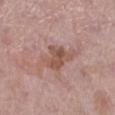The lesion was photographed on a routine skin check and not biopsied; there is no pathology result.
A close-up tile cropped from a whole-body skin photograph, about 15 mm across.
A female patient approximately 70 years of age.
On the left lower leg.
An algorithmic analysis of the crop reported an area of roughly 10 mm², an outline eccentricity of about 0.8 (0 = round, 1 = elongated), and two-axis asymmetry of about 0.3. The software also gave a within-lesion color-variation index near 3.5/10 and a peripheral color-asymmetry measure near 1. And it measured a classifier nevus-likeness of about 0/100 and a lesion-detection confidence of about 100/100.
The lesion's longest dimension is about 5 mm.
Captured under white-light illumination.